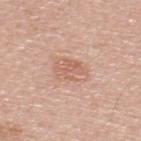Q: Was this lesion biopsied?
A: catalogued during a skin exam; not biopsied
Q: How was this image acquired?
A: 15 mm crop, total-body photography
Q: How large is the lesion?
A: about 3.5 mm
Q: Illumination type?
A: white-light
Q: Who is the patient?
A: male, aged around 65
Q: Lesion location?
A: the upper back